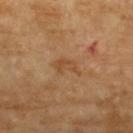Q: Is there a histopathology result?
A: imaged on a skin check; not biopsied
Q: What are the patient's age and sex?
A: female, aged 68–72
Q: Lesion size?
A: about 3 mm
Q: What is the imaging modality?
A: ~15 mm crop, total-body skin-cancer survey
Q: Illumination type?
A: cross-polarized illumination
Q: What is the anatomic site?
A: the left upper arm